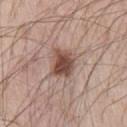Assessment:
The lesion was photographed on a routine skin check and not biopsied; there is no pathology result.
Context:
A male patient aged approximately 70. Located on the right upper arm. A 15 mm crop from a total-body photograph taken for skin-cancer surveillance.